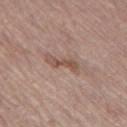| field | value |
|---|---|
| follow-up | total-body-photography surveillance lesion; no biopsy |
| patient | male, about 65 years old |
| anatomic site | the leg |
| imaging modality | ~15 mm tile from a whole-body skin photo |
| automated lesion analysis | a footprint of about 5 mm² and an eccentricity of roughly 0.95; a nevus-likeness score of about 0/100 and a detector confidence of about 95 out of 100 that the crop contains a lesion |
| lesion size | about 4 mm |
| lighting | white-light illumination |